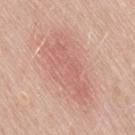<lesion>
<biopsy_status>not biopsied; imaged during a skin examination</biopsy_status>
<site>leg</site>
<patient>
  <sex>female</sex>
  <age_approx>60</age_approx>
</patient>
<lighting>white-light</lighting>
<automated_metrics>
  <nevus_likeness_0_100>45</nevus_likeness_0_100>
</automated_metrics>
<lesion_size>
  <long_diameter_mm_approx>9.0</long_diameter_mm_approx>
</lesion_size>
<image>
  <source>total-body photography crop</source>
  <field_of_view_mm>15</field_of_view_mm>
</image>
</lesion>